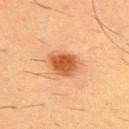Q: Was this lesion biopsied?
A: total-body-photography surveillance lesion; no biopsy
Q: Lesion location?
A: the front of the torso
Q: What kind of image is this?
A: ~15 mm tile from a whole-body skin photo
Q: Patient demographics?
A: male, about 55 years old
Q: How large is the lesion?
A: ~4 mm (longest diameter)